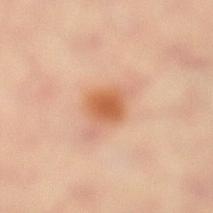Part of a total-body skin-imaging series; this lesion was reviewed on a skin check and was not flagged for biopsy. This is a cross-polarized tile. A female subject, about 40 years old. A region of skin cropped from a whole-body photographic capture, roughly 15 mm wide. From the right leg. Measured at roughly 3 mm in maximum diameter.